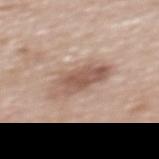Notes:
• biopsy status: imaged on a skin check; not biopsied
• automated metrics: an eccentricity of roughly 0.85 and a symmetry-axis asymmetry near 0.25; a lesion color around L≈56 a*≈18 b*≈26 in CIELAB, a lesion–skin lightness drop of about 11, and a normalized lesion–skin contrast near 7.5; a border-irregularity index near 3/10, internal color variation of about 5.5 on a 0–10 scale, and a peripheral color-asymmetry measure near 2
• acquisition: ~15 mm tile from a whole-body skin photo
• anatomic site: the upper back
• patient: male, about 70 years old
• tile lighting: white-light illumination